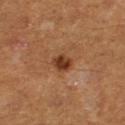Q: Is there a histopathology result?
A: imaged on a skin check; not biopsied